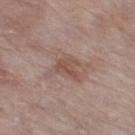Findings:
- workup: catalogued during a skin exam; not biopsied
- diameter: about 3 mm
- TBP lesion metrics: an eccentricity of roughly 0.75; an average lesion color of about L≈50 a*≈19 b*≈25 (CIELAB), a lesion–skin lightness drop of about 8, and a normalized lesion–skin contrast near 6.5; a border-irregularity index near 5.5/10, a color-variation rating of about 2/10, and a peripheral color-asymmetry measure near 1
- patient: female, aged 83 or older
- location: the right thigh
- tile lighting: white-light illumination
- image source: ~15 mm crop, total-body skin-cancer survey